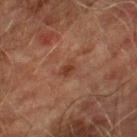No biopsy was performed on this lesion — it was imaged during a full skin examination and was not determined to be concerning. The patient is a male in their mid-70s. Cropped from a whole-body photographic skin survey; the tile spans about 15 mm. On the right lower leg.